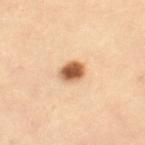This is a cross-polarized tile.
Automated image analysis of the tile measured an area of roughly 5.5 mm² and an outline eccentricity of about 0.65 (0 = round, 1 = elongated). The analysis additionally found border irregularity of about 1 on a 0–10 scale and internal color variation of about 4.5 on a 0–10 scale.
Longest diameter approximately 3 mm.
A roughly 15 mm field-of-view crop from a total-body skin photograph.
On the leg.
A female patient, roughly 45 years of age.